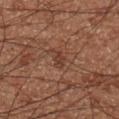Clinical impression:
Imaged during a routine full-body skin examination; the lesion was not biopsied and no histopathology is available.
Acquisition and patient details:
Automated tile analysis of the lesion measured an average lesion color of about L≈34 a*≈19 b*≈25 (CIELAB) and a normalized lesion–skin contrast near 6.5. Imaged with cross-polarized lighting. From the left lower leg. Longest diameter approximately 2.5 mm. A male patient in their 60s. A 15 mm crop from a total-body photograph taken for skin-cancer surveillance.